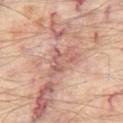Imaged during a routine full-body skin examination; the lesion was not biopsied and no histopathology is available. On the leg. A subject roughly 55 years of age. A 15 mm close-up extracted from a 3D total-body photography capture. This is a cross-polarized tile. Approximately 4 mm at its widest.